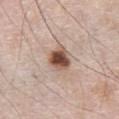* biopsy status: total-body-photography surveillance lesion; no biopsy
* image source: ~15 mm crop, total-body skin-cancer survey
* lighting: white-light
* lesion size: ≈3 mm
* subject: male, in their 80s
* location: the abdomen